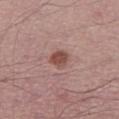Context:
The subject is a male aged 58–62. From the left lower leg. The tile uses white-light illumination. About 2.5 mm across. Cropped from a whole-body photographic skin survey; the tile spans about 15 mm.